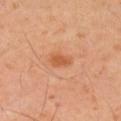notes — imaged on a skin check; not biopsied | diameter — ~2.5 mm (longest diameter) | acquisition — ~15 mm crop, total-body skin-cancer survey | patient — male, aged approximately 45 | anatomic site — the right upper arm | TBP lesion metrics — an area of roughly 4 mm², an outline eccentricity of about 0.75 (0 = round, 1 = elongated), and a symmetry-axis asymmetry near 0.25; a mean CIELAB color near L≈56 a*≈27 b*≈39, a lesion–skin lightness drop of about 9, and a normalized lesion–skin contrast near 7.